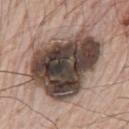Recorded during total-body skin imaging; not selected for excision or biopsy. On the chest. Longest diameter approximately 10 mm. A male patient, about 80 years old. Imaged with white-light lighting. This image is a 15 mm lesion crop taken from a total-body photograph.